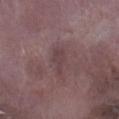Q: What are the patient's age and sex?
A: male, about 75 years old
Q: Where on the body is the lesion?
A: the right lower leg
Q: What is the imaging modality?
A: 15 mm crop, total-body photography